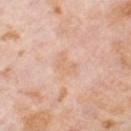Captured during whole-body skin photography for melanoma surveillance; the lesion was not biopsied.